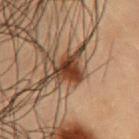{
  "biopsy_status": "not biopsied; imaged during a skin examination",
  "lighting": "cross-polarized",
  "lesion_size": {
    "long_diameter_mm_approx": 3.0
  },
  "image": {
    "source": "total-body photography crop",
    "field_of_view_mm": 15
  },
  "site": "chest",
  "automated_metrics": {
    "cielab_L": 29,
    "cielab_a": 19,
    "cielab_b": 26,
    "vs_skin_darker_L": 13.0,
    "vs_skin_contrast_norm": 12.5,
    "border_irregularity_0_10": 4.5,
    "color_variation_0_10": 4.0,
    "peripheral_color_asymmetry": 1.5
  },
  "patient": {
    "sex": "male",
    "age_approx": 55
  }
}